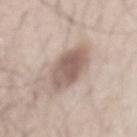lighting=white-light | anatomic site=the lower back | subject=male, about 35 years old | lesion size=~6.5 mm (longest diameter) | image=~15 mm crop, total-body skin-cancer survey | automated lesion analysis=a border-irregularity index near 2/10, internal color variation of about 4 on a 0–10 scale, and radial color variation of about 1.5; an automated nevus-likeness rating near 95 out of 100.